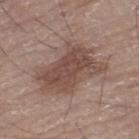{"biopsy_status": "not biopsied; imaged during a skin examination", "lighting": "white-light", "lesion_size": {"long_diameter_mm_approx": 8.5}, "patient": {"sex": "male", "age_approx": 80}, "site": "right leg", "image": {"source": "total-body photography crop", "field_of_view_mm": 15}}